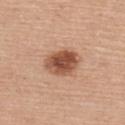notes: no biopsy performed (imaged during a skin exam) | imaging modality: 15 mm crop, total-body photography | subject: female, aged approximately 65 | body site: the back | automated metrics: a footprint of about 11 mm² and a shape eccentricity near 0.65; a lesion–skin lightness drop of about 16 and a lesion-to-skin contrast of about 10.5 (normalized; higher = more distinct); a border-irregularity index near 1.5/10, a within-lesion color-variation index near 5.5/10, and a peripheral color-asymmetry measure near 2; a nevus-likeness score of about 95/100 and a lesion-detection confidence of about 100/100 | diameter: ≈4.5 mm | illumination: white-light.